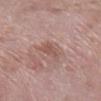Clinical impression:
The lesion was tiled from a total-body skin photograph and was not biopsied.
Acquisition and patient details:
This image is a 15 mm lesion crop taken from a total-body photograph. Automated image analysis of the tile measured two-axis asymmetry of about 0.45. It also reported roughly 8 lightness units darker than nearby skin. And it measured a border-irregularity index near 4/10, a color-variation rating of about 1/10, and a peripheral color-asymmetry measure near 0.5. On the leg. The patient is a female about 70 years old.